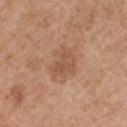{"lesion_size": {"long_diameter_mm_approx": 4.0}, "patient": {"sex": "female", "age_approx": 40}, "site": "chest", "image": {"source": "total-body photography crop", "field_of_view_mm": 15}}